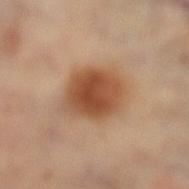Findings:
* biopsy status: catalogued during a skin exam; not biopsied
* patient: female, approximately 60 years of age
* image-analysis metrics: a border-irregularity index near 1.5/10 and peripheral color asymmetry of about 1
* diameter: ≈5.5 mm
* acquisition: ~15 mm crop, total-body skin-cancer survey
* body site: the left lower leg